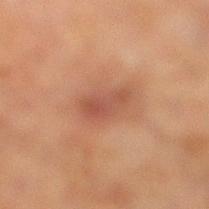  lighting: cross-polarized
  lesion_size:
    long_diameter_mm_approx: 4.0
  site: right lower leg
  patient:
    sex: male
    age_approx: 70
  image:
    source: total-body photography crop
    field_of_view_mm: 15